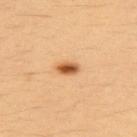The lesion was tiled from a total-body skin photograph and was not biopsied. Longest diameter approximately 2.5 mm. A female patient roughly 35 years of age. A 15 mm crop from a total-body photograph taken for skin-cancer surveillance. Automated image analysis of the tile measured an area of roughly 4 mm², a shape eccentricity near 0.8, and two-axis asymmetry of about 0.15. And it measured a border-irregularity rating of about 1/10 and internal color variation of about 4 on a 0–10 scale. It also reported an automated nevus-likeness rating near 100 out of 100 and a detector confidence of about 100 out of 100 that the crop contains a lesion. Captured under cross-polarized illumination. The lesion is located on the upper back.